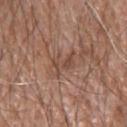This lesion was catalogued during total-body skin photography and was not selected for biopsy.
A male patient, approximately 80 years of age.
The recorded lesion diameter is about 3.5 mm.
Imaged with white-light lighting.
Located on the upper back.
Cropped from a whole-body photographic skin survey; the tile spans about 15 mm.